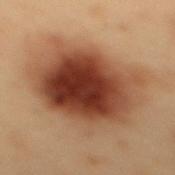workup — imaged on a skin check; not biopsied
patient — male, aged approximately 55
anatomic site — the back
illumination — cross-polarized illumination
imaging modality — total-body-photography crop, ~15 mm field of view
size — about 9.5 mm